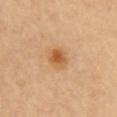notes: catalogued during a skin exam; not biopsied
lesion size: ~2.5 mm (longest diameter)
image source: ~15 mm tile from a whole-body skin photo
body site: the right upper arm
illumination: cross-polarized illumination
automated metrics: a lesion area of about 5 mm², a shape eccentricity near 0.5, and two-axis asymmetry of about 0.2; a mean CIELAB color near L≈59 a*≈24 b*≈42, roughly 10 lightness units darker than nearby skin, and a normalized lesion–skin contrast near 8; border irregularity of about 1.5 on a 0–10 scale and a color-variation rating of about 4.5/10